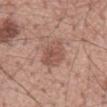{
  "biopsy_status": "not biopsied; imaged during a skin examination",
  "patient": {
    "sex": "male",
    "age_approx": 55
  },
  "lesion_size": {
    "long_diameter_mm_approx": 4.5
  },
  "automated_metrics": {
    "cielab_L": 53,
    "cielab_a": 21,
    "cielab_b": 25,
    "vs_skin_contrast_norm": 6.5,
    "nevus_likeness_0_100": 10,
    "lesion_detection_confidence_0_100": 100
  },
  "image": {
    "source": "total-body photography crop",
    "field_of_view_mm": 15
  },
  "lighting": "white-light",
  "site": "mid back"
}